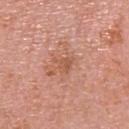Acquisition and patient details:
Imaged with white-light lighting. On the head or neck. The subject is a female in their 60s. The lesion-visualizer software estimated an area of roughly 3.5 mm², an outline eccentricity of about 0.5 (0 = round, 1 = elongated), and a shape-asymmetry score of about 0.45 (0 = symmetric). It also reported an average lesion color of about L≈56 a*≈25 b*≈32 (CIELAB) and a normalized lesion–skin contrast near 5.5. And it measured an automated nevus-likeness rating near 0 out of 100. A close-up tile cropped from a whole-body skin photograph, about 15 mm across. Measured at roughly 2.5 mm in maximum diameter.